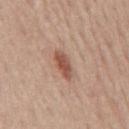Assessment:
This lesion was catalogued during total-body skin photography and was not selected for biopsy.
Background:
The patient is a male roughly 60 years of age. The lesion is on the mid back. The recorded lesion diameter is about 4 mm. Automated image analysis of the tile measured a shape eccentricity near 0.9 and two-axis asymmetry of about 0.2. The software also gave a border-irregularity index near 2.5/10, a color-variation rating of about 3.5/10, and peripheral color asymmetry of about 1. And it measured an automated nevus-likeness rating near 85 out of 100 and a lesion-detection confidence of about 100/100. Cropped from a total-body skin-imaging series; the visible field is about 15 mm.